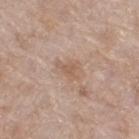follow-up: catalogued during a skin exam; not biopsied
body site: the leg
illumination: white-light illumination
subject: female, approximately 75 years of age
imaging modality: total-body-photography crop, ~15 mm field of view